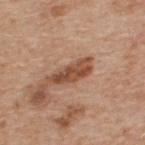Recorded during total-body skin imaging; not selected for excision or biopsy. Captured under white-light illumination. The patient is a male aged 58 to 62. Cropped from a total-body skin-imaging series; the visible field is about 15 mm. Located on the upper back. The lesion-visualizer software estimated a lesion area of about 7 mm², a shape eccentricity near 0.95, and a shape-asymmetry score of about 0.3 (0 = symmetric). The software also gave a nevus-likeness score of about 30/100 and a lesion-detection confidence of about 100/100.